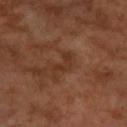biopsy status: no biopsy performed (imaged during a skin exam)
lighting: cross-polarized
lesion diameter: ~3 mm (longest diameter)
acquisition: ~15 mm tile from a whole-body skin photo
location: the arm
TBP lesion metrics: a lesion color around L≈32 a*≈21 b*≈30 in CIELAB and a normalized border contrast of about 5.5
subject: male, about 55 years old